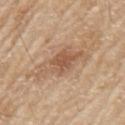Assessment:
Imaged during a routine full-body skin examination; the lesion was not biopsied and no histopathology is available.
Background:
A 15 mm close-up extracted from a 3D total-body photography capture. On the arm. A male patient, aged 78 to 82.